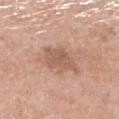workup=total-body-photography surveillance lesion; no biopsy
acquisition=15 mm crop, total-body photography
lesion diameter=≈4.5 mm
patient=female, aged approximately 70
anatomic site=the right lower leg
illumination=white-light